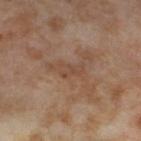{
  "biopsy_status": "not biopsied; imaged during a skin examination",
  "image": {
    "source": "total-body photography crop",
    "field_of_view_mm": 15
  },
  "lighting": "cross-polarized",
  "site": "left thigh",
  "lesion_size": {
    "long_diameter_mm_approx": 3.0
  },
  "patient": {
    "sex": "female",
    "age_approx": 55
  },
  "automated_metrics": {
    "cielab_L": 47,
    "cielab_a": 18,
    "cielab_b": 29,
    "vs_skin_darker_L": 6.0,
    "vs_skin_contrast_norm": 4.5
  }
}